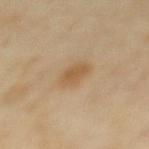Q: Illumination type?
A: cross-polarized illumination
Q: Who is the patient?
A: female, aged 43 to 47
Q: What kind of image is this?
A: total-body-photography crop, ~15 mm field of view
Q: How large is the lesion?
A: about 3 mm
Q: What is the anatomic site?
A: the back